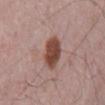site: mid back
lighting: white-light
automated_metrics:
  border_irregularity_0_10: 2.0
  color_variation_0_10: 3.0
image:
  source: total-body photography crop
  field_of_view_mm: 15
patient:
  sex: male
  age_approx: 65
lesion_size:
  long_diameter_mm_approx: 4.0